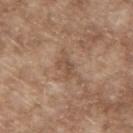Captured during whole-body skin photography for melanoma surveillance; the lesion was not biopsied. This image is a 15 mm lesion crop taken from a total-body photograph. The subject is a male in their mid- to late 60s. An algorithmic analysis of the crop reported an area of roughly 4.5 mm², an eccentricity of roughly 0.85, and a shape-asymmetry score of about 0.4 (0 = symmetric). It also reported a lesion color around L≈50 a*≈17 b*≈29 in CIELAB, about 7 CIELAB-L* units darker than the surrounding skin, and a lesion-to-skin contrast of about 5.5 (normalized; higher = more distinct). Measured at roughly 3 mm in maximum diameter. The tile uses white-light illumination. The lesion is located on the upper back.